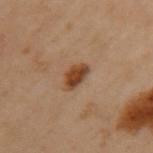Part of a total-body skin-imaging series; this lesion was reviewed on a skin check and was not flagged for biopsy. The recorded lesion diameter is about 3 mm. The patient is a female roughly 60 years of age. The tile uses cross-polarized illumination. Located on the upper back. A roughly 15 mm field-of-view crop from a total-body skin photograph.